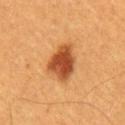Q: Is there a histopathology result?
A: total-body-photography surveillance lesion; no biopsy
Q: Lesion location?
A: the chest
Q: What is the lesion's diameter?
A: about 4.5 mm
Q: How was this image acquired?
A: 15 mm crop, total-body photography
Q: Patient demographics?
A: male, approximately 60 years of age
Q: Automated lesion metrics?
A: an eccentricity of roughly 0.7 and a symmetry-axis asymmetry near 0.25; a lesion color around L≈46 a*≈27 b*≈39 in CIELAB and roughly 16 lightness units darker than nearby skin; an automated nevus-likeness rating near 100 out of 100